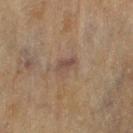Assessment:
Captured during whole-body skin photography for melanoma surveillance; the lesion was not biopsied.
Clinical summary:
The lesion is located on the right thigh. A female patient aged approximately 80. A region of skin cropped from a whole-body photographic capture, roughly 15 mm wide. The lesion-visualizer software estimated a border-irregularity rating of about 2.5/10, a within-lesion color-variation index near 4/10, and radial color variation of about 1.5. The analysis additionally found an automated nevus-likeness rating near 0 out of 100.